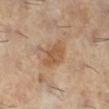  site: leg
  patient:
    sex: female
    age_approx: 60
  image:
    source: total-body photography crop
    field_of_view_mm: 15
  lighting: cross-polarized